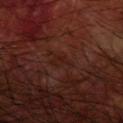Captured during whole-body skin photography for melanoma surveillance; the lesion was not biopsied.
The lesion's longest dimension is about 2.5 mm.
On the right upper arm.
A close-up tile cropped from a whole-body skin photograph, about 15 mm across.
Imaged with cross-polarized lighting.
Automated image analysis of the tile measured a mean CIELAB color near L≈20 a*≈21 b*≈21, roughly 4 lightness units darker than nearby skin, and a normalized border contrast of about 4.5. The analysis additionally found internal color variation of about 1 on a 0–10 scale and peripheral color asymmetry of about 0.5. It also reported lesion-presence confidence of about 80/100.
A male patient, roughly 60 years of age.